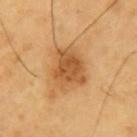  biopsy_status: not biopsied; imaged during a skin examination
  automated_metrics:
    cielab_L: 54
    cielab_a: 23
    cielab_b: 42
    vs_skin_contrast_norm: 8.0
    peripheral_color_asymmetry: 1.5
  image:
    source: total-body photography crop
    field_of_view_mm: 15
  lesion_size:
    long_diameter_mm_approx: 5.0
  lighting: cross-polarized
  site: left upper arm
  patient:
    sex: male
    age_approx: 60